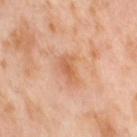Q: Was a biopsy performed?
A: catalogued during a skin exam; not biopsied
Q: Where on the body is the lesion?
A: the right thigh
Q: Illumination type?
A: cross-polarized illumination
Q: How large is the lesion?
A: about 3.5 mm
Q: Patient demographics?
A: female, in their mid- to late 50s
Q: What is the imaging modality?
A: ~15 mm crop, total-body skin-cancer survey